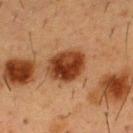biopsy_status: not biopsied; imaged during a skin examination
patient:
  sex: male
  age_approx: 55
image:
  source: total-body photography crop
  field_of_view_mm: 15
lighting: cross-polarized
site: chest